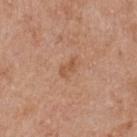This lesion was catalogued during total-body skin photography and was not selected for biopsy. A close-up tile cropped from a whole-body skin photograph, about 15 mm across. A female subject, aged 73–77. The lesion is on the upper back.